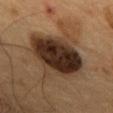Captured during whole-body skin photography for melanoma surveillance; the lesion was not biopsied. Captured under cross-polarized illumination. Automated image analysis of the tile measured an area of roughly 30 mm², an eccentricity of roughly 0.6, and a symmetry-axis asymmetry near 0.2. And it measured about 16 CIELAB-L* units darker than the surrounding skin and a lesion-to-skin contrast of about 15.5 (normalized; higher = more distinct). It also reported border irregularity of about 2.5 on a 0–10 scale and peripheral color asymmetry of about 2.5. On the upper back. A male subject aged around 55. This image is a 15 mm lesion crop taken from a total-body photograph. Measured at roughly 7 mm in maximum diameter.